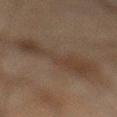<tbp_lesion>
  <automated_metrics>
    <vs_skin_darker_L>5.0</vs_skin_darker_L>
    <border_irregularity_0_10>6.0</border_irregularity_0_10>
    <color_variation_0_10>3.5</color_variation_0_10>
    <peripheral_color_asymmetry>1.0</peripheral_color_asymmetry>
    <lesion_detection_confidence_0_100>100</lesion_detection_confidence_0_100>
  </automated_metrics>
  <lighting>cross-polarized</lighting>
  <image>
    <source>total-body photography crop</source>
    <field_of_view_mm>15</field_of_view_mm>
  </image>
  <site>left lower leg</site>
  <patient>
    <sex>male</sex>
    <age_approx>45</age_approx>
  </patient>
  <lesion_size>
    <long_diameter_mm_approx>7.5</long_diameter_mm_approx>
  </lesion_size>
</tbp_lesion>